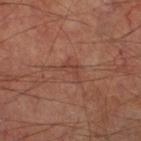This lesion was catalogued during total-body skin photography and was not selected for biopsy. The patient is a male aged around 70. Captured under cross-polarized illumination. The total-body-photography lesion software estimated a footprint of about 3 mm², a shape eccentricity near 0.85, and two-axis asymmetry of about 0.75. The lesion's longest dimension is about 3 mm. On the right lower leg. A close-up tile cropped from a whole-body skin photograph, about 15 mm across.